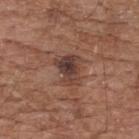Clinical impression:
Recorded during total-body skin imaging; not selected for excision or biopsy.
Clinical summary:
The tile uses white-light illumination. A male subject, aged approximately 75. Automated tile analysis of the lesion measured an eccentricity of roughly 0.7 and a symmetry-axis asymmetry near 0.4. It also reported a border-irregularity rating of about 4/10, a within-lesion color-variation index near 6.5/10, and radial color variation of about 2. Cropped from a total-body skin-imaging series; the visible field is about 15 mm. Approximately 3.5 mm at its widest. From the upper back.